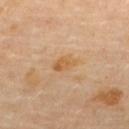This lesion was catalogued during total-body skin photography and was not selected for biopsy. A 15 mm close-up extracted from a 3D total-body photography capture. A female patient, about 60 years old. Captured under cross-polarized illumination. Longest diameter approximately 3 mm. The lesion is on the upper back.